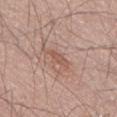Q: Was a biopsy performed?
A: imaged on a skin check; not biopsied
Q: What kind of image is this?
A: ~15 mm tile from a whole-body skin photo
Q: Who is the patient?
A: male, in their mid- to late 60s
Q: Lesion location?
A: the back
Q: What did automated image analysis measure?
A: an average lesion color of about L≈54 a*≈21 b*≈27 (CIELAB), about 7 CIELAB-L* units darker than the surrounding skin, and a lesion-to-skin contrast of about 5.5 (normalized; higher = more distinct)
Q: What is the lesion's diameter?
A: about 2.5 mm
Q: What lighting was used for the tile?
A: white-light